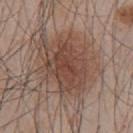Clinical summary:
This is a white-light tile. About 6 mm across. A male patient, aged approximately 45. Located on the chest. A region of skin cropped from a whole-body photographic capture, roughly 15 mm wide.